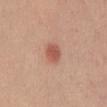lesion diameter = about 2.5 mm | location = the abdomen | subject = male, in their 30s | lighting = white-light | image = ~15 mm crop, total-body skin-cancer survey.